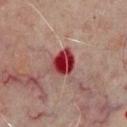biopsy_status: not biopsied; imaged during a skin examination
site: mid back
lighting: cross-polarized
lesion_size:
  long_diameter_mm_approx: 3.5
automated_metrics:
  area_mm2_approx: 8.5
  eccentricity: 0.55
  shape_asymmetry: 0.3
  border_irregularity_0_10: 3.0
  color_variation_0_10: 8.0
  peripheral_color_asymmetry: 2.5
image:
  source: total-body photography crop
  field_of_view_mm: 15
patient:
  sex: male
  age_approx: 70